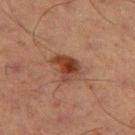Q: Was a biopsy performed?
A: no biopsy performed (imaged during a skin exam)
Q: Lesion location?
A: the right thigh
Q: What are the patient's age and sex?
A: male, roughly 65 years of age
Q: What kind of image is this?
A: total-body-photography crop, ~15 mm field of view